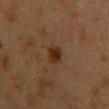image — total-body-photography crop, ~15 mm field of view
tile lighting — cross-polarized
anatomic site — the chest
diameter — about 2.5 mm
subject — female, aged around 40